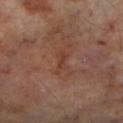  biopsy_status: not biopsied; imaged during a skin examination
  patient:
    sex: male
    age_approx: 70
  lighting: cross-polarized
  site: left lower leg
  lesion_size:
    long_diameter_mm_approx: 3.0
  image:
    source: total-body photography crop
    field_of_view_mm: 15